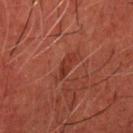<lesion>
<biopsy_status>not biopsied; imaged during a skin examination</biopsy_status>
<lesion_size>
  <long_diameter_mm_approx>4.0</long_diameter_mm_approx>
</lesion_size>
<image>
  <source>total-body photography crop</source>
  <field_of_view_mm>15</field_of_view_mm>
</image>
<patient>
  <sex>male</sex>
  <age_approx>60</age_approx>
</patient>
<site>head or neck</site>
</lesion>